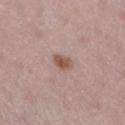A lesion tile, about 15 mm wide, cut from a 3D total-body photograph.
Imaged with white-light lighting.
The subject is a female in their mid- to late 20s.
Longest diameter approximately 2.5 mm.
Located on the leg.